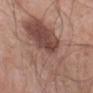Case summary:
- biopsy status: no biopsy performed (imaged during a skin exam)
- body site: the abdomen
- size: ~11.5 mm (longest diameter)
- acquisition: 15 mm crop, total-body photography
- patient: male, aged 68 to 72